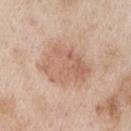biopsy status: imaged on a skin check; not biopsied | automated lesion analysis: a footprint of about 23 mm², a shape eccentricity near 0.55, and a symmetry-axis asymmetry near 0.15; a border-irregularity rating of about 2.5/10, internal color variation of about 4 on a 0–10 scale, and peripheral color asymmetry of about 1.5; a classifier nevus-likeness of about 15/100 | patient: male, in their mid-50s | image: ~15 mm crop, total-body skin-cancer survey | location: the left upper arm | tile lighting: white-light | lesion size: about 6 mm.